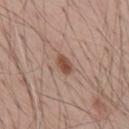biopsy status — imaged on a skin check; not biopsied | size — ~2.5 mm (longest diameter) | subject — male, aged 68 to 72 | anatomic site — the upper back | image — ~15 mm tile from a whole-body skin photo | illumination — white-light illumination.